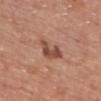Image and clinical context:
The lesion's longest dimension is about 4 mm. A 15 mm close-up extracted from a 3D total-body photography capture. This is a white-light tile. The lesion is located on the chest. A male subject, aged approximately 70. An algorithmic analysis of the crop reported an area of roughly 6.5 mm² and an outline eccentricity of about 0.8 (0 = round, 1 = elongated). The software also gave a border-irregularity rating of about 5/10, a color-variation rating of about 3.5/10, and radial color variation of about 1. And it measured a detector confidence of about 100 out of 100 that the crop contains a lesion.